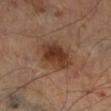| key | value |
|---|---|
| notes | total-body-photography surveillance lesion; no biopsy |